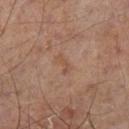biopsy status — imaged on a skin check; not biopsied